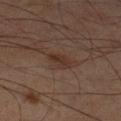| key | value |
|---|---|
| workup | total-body-photography surveillance lesion; no biopsy |
| lighting | cross-polarized |
| automated lesion analysis | border irregularity of about 3 on a 0–10 scale, internal color variation of about 1.5 on a 0–10 scale, and peripheral color asymmetry of about 0.5 |
| lesion diameter | about 3 mm |
| subject | male, about 60 years old |
| image source | ~15 mm crop, total-body skin-cancer survey |
| location | the right lower leg |